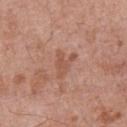Findings:
* biopsy status: imaged on a skin check; not biopsied
* automated metrics: internal color variation of about 1 on a 0–10 scale
* anatomic site: the front of the torso
* patient: male, aged 68 to 72
* tile lighting: white-light
* diameter: about 3.5 mm
* image source: ~15 mm crop, total-body skin-cancer survey